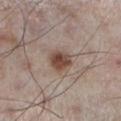Part of a total-body skin-imaging series; this lesion was reviewed on a skin check and was not flagged for biopsy.
The recorded lesion diameter is about 3 mm.
The tile uses white-light illumination.
Cropped from a whole-body photographic skin survey; the tile spans about 15 mm.
A male subject aged around 60.
From the left lower leg.
Automated tile analysis of the lesion measured an automated nevus-likeness rating near 95 out of 100 and a lesion-detection confidence of about 100/100.